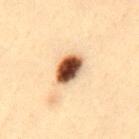Assessment: Recorded during total-body skin imaging; not selected for excision or biopsy. Acquisition and patient details: Automated image analysis of the tile measured a mean CIELAB color near L≈49 a*≈20 b*≈32 and about 25 CIELAB-L* units darker than the surrounding skin. And it measured border irregularity of about 1.5 on a 0–10 scale, a within-lesion color-variation index near 9.5/10, and peripheral color asymmetry of about 3. Captured under cross-polarized illumination. A region of skin cropped from a whole-body photographic capture, roughly 15 mm wide. The patient is a male roughly 35 years of age. Approximately 4.5 mm at its widest. Located on the mid back.